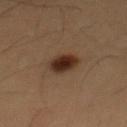Clinical impression: The lesion was photographed on a routine skin check and not biopsied; there is no pathology result. Acquisition and patient details: A 15 mm close-up extracted from a 3D total-body photography capture. The lesion's longest dimension is about 3.5 mm. A male patient aged 58–62. The lesion-visualizer software estimated a lesion area of about 7.5 mm² and a symmetry-axis asymmetry near 0.15. It also reported a mean CIELAB color near L≈29 a*≈16 b*≈25, a lesion–skin lightness drop of about 12, and a normalized lesion–skin contrast near 12. The software also gave a nevus-likeness score of about 100/100 and a lesion-detection confidence of about 100/100. The lesion is located on the abdomen.